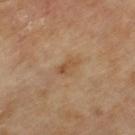The lesion was photographed on a routine skin check and not biopsied; there is no pathology result. A 15 mm crop from a total-body photograph taken for skin-cancer surveillance. The patient is a male approximately 65 years of age. The tile uses cross-polarized illumination. The recorded lesion diameter is about 3 mm.